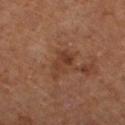The lesion was tiled from a total-body skin photograph and was not biopsied.
Approximately 4 mm at its widest.
A close-up tile cropped from a whole-body skin photograph, about 15 mm across.
The patient is a female in their mid-60s.
Captured under cross-polarized illumination.
The lesion is on the left lower leg.